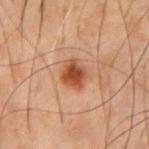workup: imaged on a skin check; not biopsied
patient: male, approximately 70 years of age
image-analysis metrics: a footprint of about 7 mm², a shape eccentricity near 0.6, and two-axis asymmetry of about 0.15; a lesion color around L≈38 a*≈20 b*≈29 in CIELAB, roughly 11 lightness units darker than nearby skin, and a lesion-to-skin contrast of about 10 (normalized; higher = more distinct); a nevus-likeness score of about 95/100 and a lesion-detection confidence of about 100/100
location: the chest
acquisition: total-body-photography crop, ~15 mm field of view
tile lighting: cross-polarized illumination